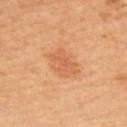Impression: Part of a total-body skin-imaging series; this lesion was reviewed on a skin check and was not flagged for biopsy. Clinical summary: Approximately 4.5 mm at its widest. A male patient, about 65 years old. The tile uses cross-polarized illumination. Located on the upper back. A 15 mm close-up extracted from a 3D total-body photography capture. Automated tile analysis of the lesion measured an area of roughly 10 mm² and a symmetry-axis asymmetry near 0.25. It also reported a classifier nevus-likeness of about 80/100 and a detector confidence of about 100 out of 100 that the crop contains a lesion.